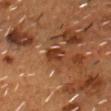Q: Was a biopsy performed?
A: imaged on a skin check; not biopsied
Q: What is the imaging modality?
A: total-body-photography crop, ~15 mm field of view
Q: Illumination type?
A: cross-polarized
Q: Automated lesion metrics?
A: a shape-asymmetry score of about 0.25 (0 = symmetric); a border-irregularity index near 2.5/10, a color-variation rating of about 5.5/10, and radial color variation of about 2.5; an automated nevus-likeness rating near 0 out of 100 and lesion-presence confidence of about 75/100
Q: What is the lesion's diameter?
A: about 2.5 mm
Q: Who is the patient?
A: male, roughly 55 years of age
Q: Lesion location?
A: the head or neck